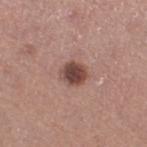<record>
<biopsy_status>not biopsied; imaged during a skin examination</biopsy_status>
<lesion_size>
  <long_diameter_mm_approx>3.0</long_diameter_mm_approx>
</lesion_size>
<image>
  <source>total-body photography crop</source>
  <field_of_view_mm>15</field_of_view_mm>
</image>
<site>right thigh</site>
<patient>
  <sex>female</sex>
  <age_approx>55</age_approx>
</patient>
</record>